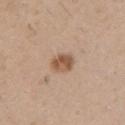biopsy_status: not biopsied; imaged during a skin examination
lesion_size:
  long_diameter_mm_approx: 2.5
lighting: white-light
automated_metrics:
  color_variation_0_10: 4.0
  peripheral_color_asymmetry: 1.5
  nevus_likeness_0_100: 90
site: chest
image:
  source: total-body photography crop
  field_of_view_mm: 15
patient:
  sex: male
  age_approx: 50